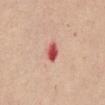Part of a total-body skin-imaging series; this lesion was reviewed on a skin check and was not flagged for biopsy.
A female subject, in their mid- to late 60s.
A 15 mm crop from a total-body photograph taken for skin-cancer surveillance.
On the abdomen.
Approximately 2.5 mm at its widest.
This is a white-light tile.